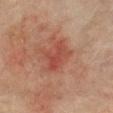  biopsy_status: not biopsied; imaged during a skin examination
  image:
    source: total-body photography crop
    field_of_view_mm: 15
  site: front of the torso
  patient:
    sex: female
    age_approx: 80
  lesion_size:
    long_diameter_mm_approx: 4.0